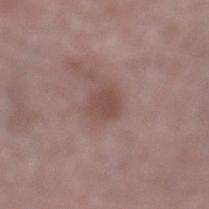Assessment: The lesion was tiled from a total-body skin photograph and was not biopsied. Image and clinical context: Measured at roughly 2.5 mm in maximum diameter. A male subject, roughly 75 years of age. Automated image analysis of the tile measured a lesion color around L≈48 a*≈19 b*≈23 in CIELAB, about 7 CIELAB-L* units darker than the surrounding skin, and a normalized lesion–skin contrast near 6. The analysis additionally found a lesion-detection confidence of about 100/100. Cropped from a total-body skin-imaging series; the visible field is about 15 mm. From the left lower leg.